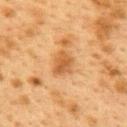Notes:
– biopsy status · total-body-photography surveillance lesion; no biopsy
– patient · female, roughly 40 years of age
– lesion diameter · ~3 mm (longest diameter)
– tile lighting · cross-polarized
– acquisition · 15 mm crop, total-body photography
– location · the upper back